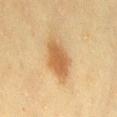follow-up = total-body-photography surveillance lesion; no biopsy
location = the lower back
acquisition = total-body-photography crop, ~15 mm field of view
tile lighting = cross-polarized
subject = female, in their mid- to late 50s